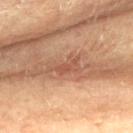Captured during whole-body skin photography for melanoma surveillance; the lesion was not biopsied. This is a cross-polarized tile. A female patient, approximately 80 years of age. A 15 mm close-up extracted from a 3D total-body photography capture. Located on the upper back. The recorded lesion diameter is about 4 mm.